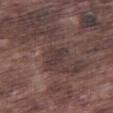biopsy status: total-body-photography surveillance lesion; no biopsy
lesion diameter: ~3 mm (longest diameter)
image: total-body-photography crop, ~15 mm field of view
body site: the right thigh
patient: male, in their mid- to late 70s
illumination: white-light illumination